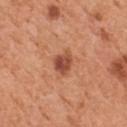Assessment:
No biopsy was performed on this lesion — it was imaged during a full skin examination and was not determined to be concerning.
Background:
Captured under white-light illumination. A 15 mm crop from a total-body photograph taken for skin-cancer surveillance. About 3 mm across. The lesion is on the chest. A male subject, aged 53 to 57.